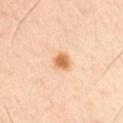Impression:
Recorded during total-body skin imaging; not selected for excision or biopsy.
Image and clinical context:
A male patient in their mid- to late 40s. Automated image analysis of the tile measured a lesion area of about 4.5 mm², an outline eccentricity of about 0.55 (0 = round, 1 = elongated), and a symmetry-axis asymmetry near 0.2. It also reported a classifier nevus-likeness of about 100/100 and lesion-presence confidence of about 100/100. A 15 mm crop from a total-body photograph taken for skin-cancer surveillance. Located on the right upper arm. Captured under cross-polarized illumination. The lesion's longest dimension is about 2.5 mm.